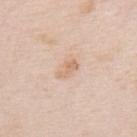<case>
  <biopsy_status>not biopsied; imaged during a skin examination</biopsy_status>
  <lighting>white-light</lighting>
  <patient>
    <sex>male</sex>
    <age_approx>75</age_approx>
  </patient>
  <lesion_size>
    <long_diameter_mm_approx>2.5</long_diameter_mm_approx>
  </lesion_size>
  <image>
    <source>total-body photography crop</source>
    <field_of_view_mm>15</field_of_view_mm>
  </image>
  <site>upper back</site>
  <automated_metrics>
    <area_mm2_approx>3.0</area_mm2_approx>
    <eccentricity>0.85</eccentricity>
    <shape_asymmetry>0.4</shape_asymmetry>
    <border_irregularity_0_10>4.5</border_irregularity_0_10>
    <color_variation_0_10>1.0</color_variation_0_10>
    <peripheral_color_asymmetry>0.5</peripheral_color_asymmetry>
  </automated_metrics>
</case>